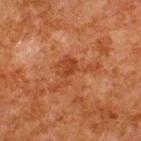Imaged during a routine full-body skin examination; the lesion was not biopsied and no histopathology is available.
A close-up tile cropped from a whole-body skin photograph, about 15 mm across.
The lesion's longest dimension is about 6 mm.
The lesion is on the back.
Imaged with cross-polarized lighting.
A male patient, about 80 years old.